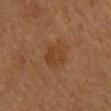workup: imaged on a skin check; not biopsied
lesion size: about 4 mm
subject: female, aged 53–57
location: the arm
imaging modality: 15 mm crop, total-body photography
lighting: cross-polarized illumination